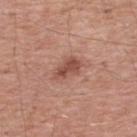Impression:
Recorded during total-body skin imaging; not selected for excision or biopsy.
Image and clinical context:
The recorded lesion diameter is about 3.5 mm. Captured under white-light illumination. A region of skin cropped from a whole-body photographic capture, roughly 15 mm wide. A male patient about 55 years old. On the upper back.